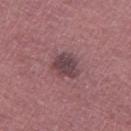The lesion was photographed on a routine skin check and not biopsied; there is no pathology result. The lesion-visualizer software estimated a lesion area of about 8 mm², a shape eccentricity near 0.55, and a symmetry-axis asymmetry near 0.2. The analysis additionally found border irregularity of about 2 on a 0–10 scale and a within-lesion color-variation index near 3/10. The lesion is on the left thigh. A male patient, about 60 years old. This is a white-light tile. A roughly 15 mm field-of-view crop from a total-body skin photograph. Longest diameter approximately 3.5 mm.